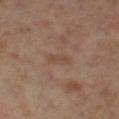<lesion>
  <biopsy_status>not biopsied; imaged during a skin examination</biopsy_status>
  <patient>
    <sex>female</sex>
    <age_approx>55</age_approx>
  </patient>
  <automated_metrics>
    <area_mm2_approx>2.5</area_mm2_approx>
    <eccentricity>0.9</eccentricity>
    <shape_asymmetry>0.25</shape_asymmetry>
    <vs_skin_darker_L>6.0</vs_skin_darker_L>
    <vs_skin_contrast_norm>5.0</vs_skin_contrast_norm>
  </automated_metrics>
  <lesion_size>
    <long_diameter_mm_approx>2.5</long_diameter_mm_approx>
  </lesion_size>
  <image>
    <source>total-body photography crop</source>
    <field_of_view_mm>15</field_of_view_mm>
  </image>
  <site>leg</site>
  <lighting>cross-polarized</lighting>
</lesion>